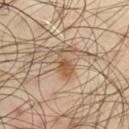Q: Was a biopsy performed?
A: no biopsy performed (imaged during a skin exam)
Q: What is the imaging modality?
A: total-body-photography crop, ~15 mm field of view
Q: Patient demographics?
A: male, approximately 45 years of age
Q: Lesion location?
A: the front of the torso